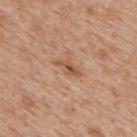Q: Was a biopsy performed?
A: total-body-photography surveillance lesion; no biopsy